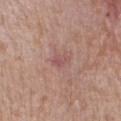follow-up=catalogued during a skin exam; not biopsied
body site=the right forearm
lesion size=≈3 mm
subject=male, aged around 55
TBP lesion metrics=a mean CIELAB color near L≈53 a*≈23 b*≈22, roughly 7 lightness units darker than nearby skin, and a normalized border contrast of about 5
image source=~15 mm crop, total-body skin-cancer survey